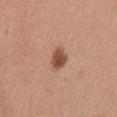The lesion was tiled from a total-body skin photograph and was not biopsied. On the lower back. A roughly 15 mm field-of-view crop from a total-body skin photograph. The patient is a female aged around 30. Automated tile analysis of the lesion measured a border-irregularity rating of about 2.5/10 and a within-lesion color-variation index near 3/10. And it measured a nevus-likeness score of about 100/100 and lesion-presence confidence of about 100/100. Measured at roughly 3 mm in maximum diameter. Captured under white-light illumination.